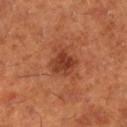Q: Was this lesion biopsied?
A: total-body-photography surveillance lesion; no biopsy
Q: Where on the body is the lesion?
A: the right lower leg
Q: What are the patient's age and sex?
A: male, approximately 65 years of age
Q: Lesion size?
A: about 3 mm
Q: What did automated image analysis measure?
A: border irregularity of about 2.5 on a 0–10 scale and radial color variation of about 1.5; a nevus-likeness score of about 85/100 and a detector confidence of about 100 out of 100 that the crop contains a lesion
Q: How was the tile lit?
A: cross-polarized
Q: What is the imaging modality?
A: 15 mm crop, total-body photography